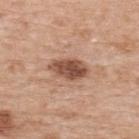biopsy status = no biopsy performed (imaged during a skin exam) | subject = male, about 70 years old | size = about 4.5 mm | illumination = white-light illumination | site = the upper back | TBP lesion metrics = internal color variation of about 5 on a 0–10 scale and a peripheral color-asymmetry measure near 2 | image = total-body-photography crop, ~15 mm field of view.